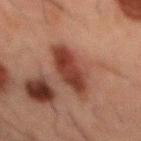Q: Was a biopsy performed?
A: total-body-photography surveillance lesion; no biopsy
Q: Lesion size?
A: ~6.5 mm (longest diameter)
Q: Lesion location?
A: the mid back
Q: How was this image acquired?
A: ~15 mm crop, total-body skin-cancer survey
Q: What are the patient's age and sex?
A: male, aged 48 to 52